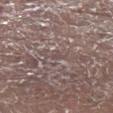Case summary:
* anatomic site · the left lower leg
* tile lighting · white-light
* patient · male, about 70 years old
* acquisition · ~15 mm tile from a whole-body skin photo
* biopsy diagnosis · an invasive squamous cell carcinoma (malignant)